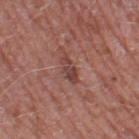Imaged during a routine full-body skin examination; the lesion was not biopsied and no histopathology is available. The lesion-visualizer software estimated a lesion area of about 4 mm² and a symmetry-axis asymmetry near 0.3. And it measured a lesion color around L≈43 a*≈23 b*≈23 in CIELAB, roughly 9 lightness units darker than nearby skin, and a normalized lesion–skin contrast near 7.5. From the right thigh. A 15 mm close-up extracted from a 3D total-body photography capture. The patient is a male aged 58–62. About 3.5 mm across.